The lesion-visualizer software estimated a lesion-to-skin contrast of about 8.5 (normalized; higher = more distinct). The software also gave a border-irregularity index near 2.5/10, a within-lesion color-variation index near 9/10, and radial color variation of about 3.5. It also reported a nevus-likeness score of about 65/100 and a detector confidence of about 100 out of 100 that the crop contains a lesion. Measured at roughly 11 mm in maximum diameter. On the leg. The subject is a female in their mid- to late 60s. The tile uses white-light illumination. A lesion tile, about 15 mm wide, cut from a 3D total-body photograph: 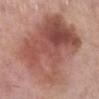Q: What did the biopsy show?
A: an invasive melanoma, superficial spreading type (Breslow thickness 0.2 mm, mitotic rate <1/mm²)